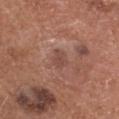This lesion was catalogued during total-body skin photography and was not selected for biopsy.
From the head or neck.
A male subject, roughly 60 years of age.
A 15 mm close-up extracted from a 3D total-body photography capture.